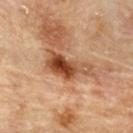Clinical summary:
The lesion is on the upper back. Cropped from a total-body skin-imaging series; the visible field is about 15 mm. The total-body-photography lesion software estimated a footprint of about 10 mm² and a shape-asymmetry score of about 0.4 (0 = symmetric). The software also gave a lesion color around L≈46 a*≈25 b*≈35 in CIELAB, a lesion–skin lightness drop of about 16, and a lesion-to-skin contrast of about 11 (normalized; higher = more distinct). The software also gave a border-irregularity rating of about 5/10 and internal color variation of about 7.5 on a 0–10 scale. And it measured lesion-presence confidence of about 100/100. Imaged with cross-polarized lighting. A male patient, aged 83 or older. The recorded lesion diameter is about 5 mm.